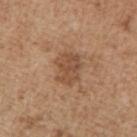Impression: Part of a total-body skin-imaging series; this lesion was reviewed on a skin check and was not flagged for biopsy. Clinical summary: An algorithmic analysis of the crop reported radial color variation of about 1. The subject is a male aged 63 to 67. This is a white-light tile. The recorded lesion diameter is about 4 mm. Located on the arm. Cropped from a whole-body photographic skin survey; the tile spans about 15 mm.